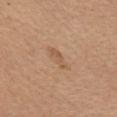follow-up: catalogued during a skin exam; not biopsied
image source: ~15 mm tile from a whole-body skin photo
anatomic site: the chest
tile lighting: white-light illumination
patient: female, approximately 65 years of age
lesion diameter: ~3 mm (longest diameter)